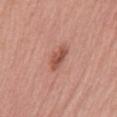Imaged during a routine full-body skin examination; the lesion was not biopsied and no histopathology is available.
This is a white-light tile.
The lesion is located on the lower back.
A lesion tile, about 15 mm wide, cut from a 3D total-body photograph.
A male subject in their 70s.
The lesion's longest dimension is about 3 mm.